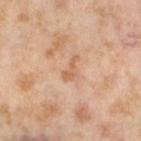Assessment:
Captured during whole-body skin photography for melanoma surveillance; the lesion was not biopsied.
Image and clinical context:
This is a cross-polarized tile. Located on the leg. A roughly 15 mm field-of-view crop from a total-body skin photograph. About 3 mm across. Automated tile analysis of the lesion measured an area of roughly 2.5 mm², an outline eccentricity of about 0.9 (0 = round, 1 = elongated), and a shape-asymmetry score of about 0.55 (0 = symmetric). A female subject in their mid-50s.